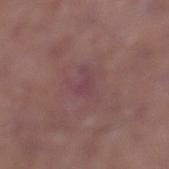Q: Is there a histopathology result?
A: no biopsy performed (imaged during a skin exam)
Q: Patient demographics?
A: male, aged around 65
Q: What did automated image analysis measure?
A: an area of roughly 3 mm², a shape eccentricity near 0.7, and two-axis asymmetry of about 0.55; a lesion color around L≈42 a*≈23 b*≈14 in CIELAB, about 5 CIELAB-L* units darker than the surrounding skin, and a normalized lesion–skin contrast near 5; a color-variation rating of about 0.5/10 and a peripheral color-asymmetry measure near 0; an automated nevus-likeness rating near 0 out of 100
Q: How was this image acquired?
A: ~15 mm tile from a whole-body skin photo
Q: How was the tile lit?
A: white-light
Q: Lesion location?
A: the left lower leg
Q: What is the lesion's diameter?
A: about 2.5 mm